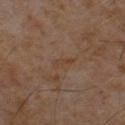Clinical impression:
This lesion was catalogued during total-body skin photography and was not selected for biopsy.
Clinical summary:
Cropped from a whole-body photographic skin survey; the tile spans about 15 mm. Approximately 2.5 mm at its widest. On the chest. A male patient in their 60s. Imaged with cross-polarized lighting.